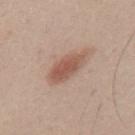Recorded during total-body skin imaging; not selected for excision or biopsy.
Automated tile analysis of the lesion measured roughly 11 lightness units darker than nearby skin and a lesion-to-skin contrast of about 7.5 (normalized; higher = more distinct). And it measured border irregularity of about 2.5 on a 0–10 scale, a within-lesion color-variation index near 3/10, and a peripheral color-asymmetry measure near 1.
This is a white-light tile.
From the mid back.
A 15 mm crop from a total-body photograph taken for skin-cancer surveillance.
Longest diameter approximately 5.5 mm.
A male subject, in their 40s.